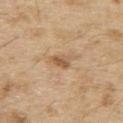Q: Is there a histopathology result?
A: catalogued during a skin exam; not biopsied
Q: What is the anatomic site?
A: the upper back
Q: How was this image acquired?
A: total-body-photography crop, ~15 mm field of view
Q: How was the tile lit?
A: white-light
Q: What did automated image analysis measure?
A: a mean CIELAB color near L≈57 a*≈18 b*≈36, roughly 10 lightness units darker than nearby skin, and a normalized border contrast of about 7; border irregularity of about 3 on a 0–10 scale, internal color variation of about 4 on a 0–10 scale, and radial color variation of about 1.5
Q: Who is the patient?
A: male, in their 70s
Q: What is the lesion's diameter?
A: ≈2.5 mm